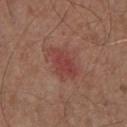Q: Was this lesion biopsied?
A: catalogued during a skin exam; not biopsied
Q: What is the lesion's diameter?
A: ≈4.5 mm
Q: What kind of image is this?
A: ~15 mm crop, total-body skin-cancer survey
Q: What did automated image analysis measure?
A: a symmetry-axis asymmetry near 0.3; a lesion color around L≈43 a*≈26 b*≈24 in CIELAB, roughly 7 lightness units darker than nearby skin, and a normalized border contrast of about 6; border irregularity of about 3.5 on a 0–10 scale, a color-variation rating of about 2.5/10, and peripheral color asymmetry of about 1
Q: What is the anatomic site?
A: the chest
Q: What are the patient's age and sex?
A: male, about 55 years old
Q: What lighting was used for the tile?
A: white-light